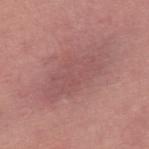Q: Was a biopsy performed?
A: total-body-photography surveillance lesion; no biopsy
Q: What is the anatomic site?
A: the upper back
Q: Illumination type?
A: white-light illumination
Q: What is the imaging modality?
A: ~15 mm tile from a whole-body skin photo
Q: Patient demographics?
A: male, aged 53 to 57
Q: What is the lesion's diameter?
A: ~8 mm (longest diameter)
Q: Automated lesion metrics?
A: a lesion color around L≈52 a*≈24 b*≈21 in CIELAB and a normalized border contrast of about 5; a border-irregularity rating of about 4.5/10, a within-lesion color-variation index near 2.5/10, and radial color variation of about 1; a nevus-likeness score of about 0/100 and a detector confidence of about 55 out of 100 that the crop contains a lesion